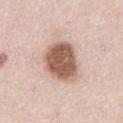Q: Is there a histopathology result?
A: catalogued during a skin exam; not biopsied